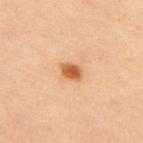Impression: Captured during whole-body skin photography for melanoma surveillance; the lesion was not biopsied. Acquisition and patient details: Imaged with cross-polarized lighting. From the upper back. A lesion tile, about 15 mm wide, cut from a 3D total-body photograph. The lesion's longest dimension is about 2.5 mm. A female patient approximately 65 years of age.